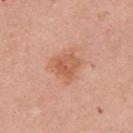biopsy_status: not biopsied; imaged during a skin examination
site: right upper arm
patient:
  sex: female
  age_approx: 65
image:
  source: total-body photography crop
  field_of_view_mm: 15